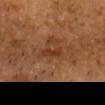Clinical summary: The lesion is located on the front of the torso. A male patient aged around 60. A region of skin cropped from a whole-body photographic capture, roughly 15 mm wide. Measured at roughly 2.5 mm in maximum diameter. Automated tile analysis of the lesion measured a lesion area of about 3 mm², a shape eccentricity near 0.85, and a shape-asymmetry score of about 0.25 (0 = symmetric). The analysis additionally found about 7 CIELAB-L* units darker than the surrounding skin. The software also gave a nevus-likeness score of about 0/100.